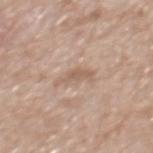Part of a total-body skin-imaging series; this lesion was reviewed on a skin check and was not flagged for biopsy. A male patient, in their mid-60s. The lesion is on the mid back. Cropped from a whole-body photographic skin survey; the tile spans about 15 mm.